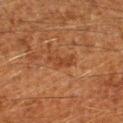Q: Was a biopsy performed?
A: no biopsy performed (imaged during a skin exam)
Q: What is the anatomic site?
A: the left forearm
Q: What kind of image is this?
A: 15 mm crop, total-body photography
Q: Who is the patient?
A: male, aged approximately 60
Q: Automated lesion metrics?
A: a lesion color around L≈36 a*≈22 b*≈32 in CIELAB, roughly 6 lightness units darker than nearby skin, and a normalized border contrast of about 5.5; a border-irregularity rating of about 5/10, a within-lesion color-variation index near 1/10, and peripheral color asymmetry of about 0; a classifier nevus-likeness of about 0/100 and lesion-presence confidence of about 100/100
Q: What lighting was used for the tile?
A: cross-polarized illumination
Q: Lesion size?
A: ≈3 mm